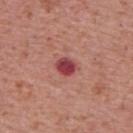The lesion was tiled from a total-body skin photograph and was not biopsied. The total-body-photography lesion software estimated a footprint of about 4 mm². The software also gave a mean CIELAB color near L≈43 a*≈34 b*≈22, roughly 15 lightness units darker than nearby skin, and a normalized lesion–skin contrast near 11.5. And it measured border irregularity of about 2 on a 0–10 scale and a within-lesion color-variation index near 4/10. It also reported a nevus-likeness score of about 0/100 and a detector confidence of about 100 out of 100 that the crop contains a lesion. This is a white-light tile. Approximately 2.5 mm at its widest. A close-up tile cropped from a whole-body skin photograph, about 15 mm across. The subject is a male in their 70s. The lesion is located on the upper back.